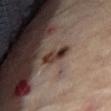Clinical summary:
Longest diameter approximately 3.5 mm. The total-body-photography lesion software estimated a footprint of about 4 mm², a shape eccentricity near 0.95, and a shape-asymmetry score of about 0.3 (0 = symmetric). The software also gave an average lesion color of about L≈33 a*≈17 b*≈23 (CIELAB), a lesion–skin lightness drop of about 12, and a lesion-to-skin contrast of about 11 (normalized; higher = more distinct). The analysis additionally found a classifier nevus-likeness of about 75/100 and a detector confidence of about 60 out of 100 that the crop contains a lesion. A 15 mm crop from a total-body photograph taken for skin-cancer surveillance. A female subject aged approximately 60. Located on the abdomen. This is a cross-polarized tile.